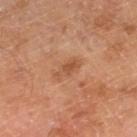The lesion was tiled from a total-body skin photograph and was not biopsied. A male subject in their mid- to late 60s. Located on the right lower leg. A lesion tile, about 15 mm wide, cut from a 3D total-body photograph.